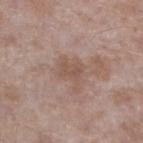This lesion was catalogued during total-body skin photography and was not selected for biopsy.
The patient is a male in their mid- to late 40s.
From the left lower leg.
A close-up tile cropped from a whole-body skin photograph, about 15 mm across.
Imaged with white-light lighting.
Measured at roughly 3.5 mm in maximum diameter.